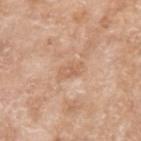Clinical impression: Part of a total-body skin-imaging series; this lesion was reviewed on a skin check and was not flagged for biopsy. Context: On the arm. A 15 mm crop from a total-body photograph taken for skin-cancer surveillance. The recorded lesion diameter is about 3 mm. A female subject roughly 75 years of age. An algorithmic analysis of the crop reported a mean CIELAB color near L≈62 a*≈20 b*≈33 and a lesion-to-skin contrast of about 5 (normalized; higher = more distinct). And it measured border irregularity of about 3 on a 0–10 scale. Imaged with white-light lighting.